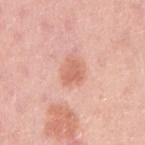Findings:
- image — 15 mm crop, total-body photography
- location — the right upper arm
- lesion size — ≈3 mm
- lighting — white-light
- subject — female, aged around 30